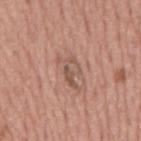Impression:
Captured during whole-body skin photography for melanoma surveillance; the lesion was not biopsied.
Background:
About 4 mm across. From the mid back. Cropped from a total-body skin-imaging series; the visible field is about 15 mm. A male subject in their mid-70s.